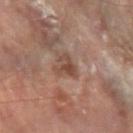Part of a total-body skin-imaging series; this lesion was reviewed on a skin check and was not flagged for biopsy. A 15 mm close-up tile from a total-body photography series done for melanoma screening. Measured at roughly 3 mm in maximum diameter. The subject is a male about 85 years old or older. From the left forearm.